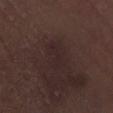The total-body-photography lesion software estimated a lesion area of about 5.5 mm² and an eccentricity of roughly 0.9. The software also gave border irregularity of about 5 on a 0–10 scale, a within-lesion color-variation index near 1/10, and a peripheral color-asymmetry measure near 0.5. The analysis additionally found an automated nevus-likeness rating near 0 out of 100 and a detector confidence of about 85 out of 100 that the crop contains a lesion.
Cropped from a total-body skin-imaging series; the visible field is about 15 mm.
A male subject, aged 68–72.
From the left lower leg.
This is a white-light tile.
Approximately 3.5 mm at its widest.